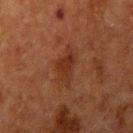Assessment:
This lesion was catalogued during total-body skin photography and was not selected for biopsy.
Acquisition and patient details:
A male patient in their 60s. The recorded lesion diameter is about 4 mm. A 15 mm close-up tile from a total-body photography series done for melanoma screening. The lesion-visualizer software estimated an average lesion color of about L≈25 a*≈20 b*≈26 (CIELAB), a lesion–skin lightness drop of about 6, and a normalized lesion–skin contrast near 6.5. The software also gave a border-irregularity rating of about 5/10, a color-variation rating of about 2.5/10, and a peripheral color-asymmetry measure near 1. And it measured a nevus-likeness score of about 5/100. On the right upper arm.